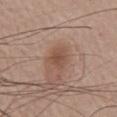biopsy status — catalogued during a skin exam; not biopsied | subject — male, aged approximately 75 | location — the chest | acquisition — total-body-photography crop, ~15 mm field of view | lesion size — ~3 mm (longest diameter) | TBP lesion metrics — a lesion area of about 4.5 mm², an eccentricity of roughly 0.7, and a shape-asymmetry score of about 0.3 (0 = symmetric); a mean CIELAB color near L≈49 a*≈19 b*≈27, roughly 9 lightness units darker than nearby skin, and a normalized lesion–skin contrast near 7; an automated nevus-likeness rating near 25 out of 100 and lesion-presence confidence of about 100/100 | lighting — white-light.